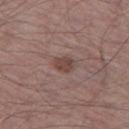The lesion was tiled from a total-body skin photograph and was not biopsied.
A roughly 15 mm field-of-view crop from a total-body skin photograph.
Imaged with white-light lighting.
Measured at roughly 2.5 mm in maximum diameter.
Located on the left thigh.
A male subject, in their mid- to late 60s.